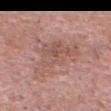Notes:
• notes: imaged on a skin check; not biopsied
• imaging modality: ~15 mm tile from a whole-body skin photo
• lighting: white-light
• size: ~6.5 mm (longest diameter)
• automated lesion analysis: a symmetry-axis asymmetry near 0.5; a mean CIELAB color near L≈54 a*≈21 b*≈25, a lesion–skin lightness drop of about 7, and a lesion-to-skin contrast of about 5.5 (normalized; higher = more distinct); a border-irregularity index near 7/10 and a peripheral color-asymmetry measure near 1.5; an automated nevus-likeness rating near 0 out of 100
• subject: male, roughly 55 years of age